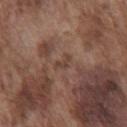Assessment:
Imaged during a routine full-body skin examination; the lesion was not biopsied and no histopathology is available.
Background:
Captured under white-light illumination. The subject is a male aged 73–77. Measured at roughly 2.5 mm in maximum diameter. The lesion is on the chest. A 15 mm crop from a total-body photograph taken for skin-cancer surveillance.